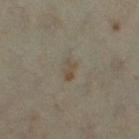Located on the left thigh.
The lesion-visualizer software estimated about 6 CIELAB-L* units darker than the surrounding skin and a normalized border contrast of about 6.
Captured under cross-polarized illumination.
A 15 mm close-up tile from a total-body photography series done for melanoma screening.
About 2.5 mm across.
The subject is a female in their mid-30s.